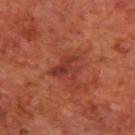Impression:
This lesion was catalogued during total-body skin photography and was not selected for biopsy.
Background:
A male subject aged 68–72. The recorded lesion diameter is about 3.5 mm. The lesion is located on the upper back. Captured under cross-polarized illumination. A roughly 15 mm field-of-view crop from a total-body skin photograph.